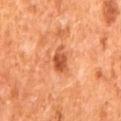• biopsy status — no biopsy performed (imaged during a skin exam)
• imaging modality — 15 mm crop, total-body photography
• subject — male, aged 63–67
• TBP lesion metrics — an automated nevus-likeness rating near 60 out of 100 and lesion-presence confidence of about 100/100
• illumination — cross-polarized illumination
• site — the back
• lesion diameter — about 3.5 mm